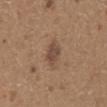Q: Was a biopsy performed?
A: total-body-photography surveillance lesion; no biopsy
Q: What lighting was used for the tile?
A: white-light
Q: What are the patient's age and sex?
A: male, approximately 65 years of age
Q: What is the imaging modality?
A: ~15 mm tile from a whole-body skin photo
Q: What is the anatomic site?
A: the abdomen
Q: Automated lesion metrics?
A: a footprint of about 5 mm², a shape eccentricity near 0.75, and a shape-asymmetry score of about 0.25 (0 = symmetric); a lesion color around L≈46 a*≈17 b*≈27 in CIELAB, about 9 CIELAB-L* units darker than the surrounding skin, and a normalized border contrast of about 7.5; a border-irregularity rating of about 2.5/10, a color-variation rating of about 2/10, and a peripheral color-asymmetry measure near 0.5; an automated nevus-likeness rating near 50 out of 100 and lesion-presence confidence of about 100/100